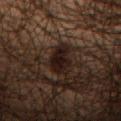| field | value |
|---|---|
| follow-up | imaged on a skin check; not biopsied |
| body site | the leg |
| patient | male, aged 48–52 |
| image source | 15 mm crop, total-body photography |